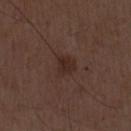{"biopsy_status": "not biopsied; imaged during a skin examination", "patient": {"sex": "male", "age_approx": 50}, "image": {"source": "total-body photography crop", "field_of_view_mm": 15}, "lighting": "white-light", "lesion_size": {"long_diameter_mm_approx": 2.5}, "automated_metrics": {"area_mm2_approx": 4.5, "eccentricity": 0.55, "shape_asymmetry": 0.3, "border_irregularity_0_10": 3.0, "color_variation_0_10": 2.0}}